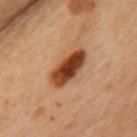Part of a total-body skin-imaging series; this lesion was reviewed on a skin check and was not flagged for biopsy.